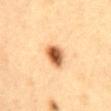Image and clinical context:
A 15 mm close-up extracted from a 3D total-body photography capture. The lesion is on the chest. The tile uses cross-polarized illumination. A female patient, aged 28 to 32. The recorded lesion diameter is about 3 mm.